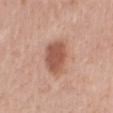Q: Was a biopsy performed?
A: catalogued during a skin exam; not biopsied
Q: What is the anatomic site?
A: the back
Q: Who is the patient?
A: female, aged approximately 55
Q: What kind of image is this?
A: ~15 mm crop, total-body skin-cancer survey